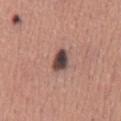Q: Was a biopsy performed?
A: catalogued during a skin exam; not biopsied
Q: Patient demographics?
A: male, about 45 years old
Q: Lesion location?
A: the chest
Q: What lighting was used for the tile?
A: white-light illumination
Q: What kind of image is this?
A: ~15 mm tile from a whole-body skin photo
Q: How large is the lesion?
A: about 3 mm